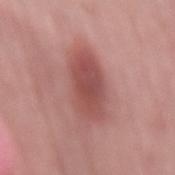Part of a total-body skin-imaging series; this lesion was reviewed on a skin check and was not flagged for biopsy. About 7 mm across. Automated image analysis of the tile measured an area of roughly 18 mm² and a symmetry-axis asymmetry near 0.15. And it measured a lesion–skin lightness drop of about 11. The software also gave a nevus-likeness score of about 80/100. On the lower back. A 15 mm crop from a total-body photograph taken for skin-cancer surveillance. A male subject aged 53–57.